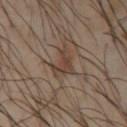This lesion was catalogued during total-body skin photography and was not selected for biopsy.
The subject is a male about 40 years old.
The tile uses cross-polarized illumination.
From the left upper arm.
A 15 mm crop from a total-body photograph taken for skin-cancer surveillance.
Measured at roughly 3.5 mm in maximum diameter.